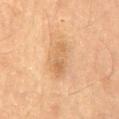No biopsy was performed on this lesion — it was imaged during a full skin examination and was not determined to be concerning. Imaged with cross-polarized lighting. A male patient, about 60 years old. Automated tile analysis of the lesion measured about 8 CIELAB-L* units darker than the surrounding skin and a normalized lesion–skin contrast near 6. And it measured border irregularity of about 3 on a 0–10 scale, a within-lesion color-variation index near 3.5/10, and radial color variation of about 1. And it measured a nevus-likeness score of about 5/100 and a lesion-detection confidence of about 100/100. Approximately 4 mm at its widest. A close-up tile cropped from a whole-body skin photograph, about 15 mm across.